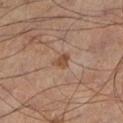| key | value |
|---|---|
| workup | total-body-photography surveillance lesion; no biopsy |
| image-analysis metrics | an area of roughly 3.5 mm², an outline eccentricity of about 0.65 (0 = round, 1 = elongated), and a shape-asymmetry score of about 0.45 (0 = symmetric); an average lesion color of about L≈45 a*≈18 b*≈29 (CIELAB) and a lesion–skin lightness drop of about 8; a border-irregularity rating of about 4/10, a color-variation rating of about 1.5/10, and a peripheral color-asymmetry measure near 0.5; a classifier nevus-likeness of about 30/100 and lesion-presence confidence of about 100/100 |
| lesion size | ~2.5 mm (longest diameter) |
| patient | male, about 55 years old |
| body site | the left lower leg |
| tile lighting | cross-polarized illumination |
| image | ~15 mm crop, total-body skin-cancer survey |